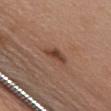{
  "biopsy_status": "not biopsied; imaged during a skin examination",
  "image": {
    "source": "total-body photography crop",
    "field_of_view_mm": 15
  },
  "site": "chest",
  "patient": {
    "sex": "male",
    "age_approx": 60
  },
  "automated_metrics": {
    "area_mm2_approx": 3.5,
    "eccentricity": 0.85,
    "shape_asymmetry": 0.4,
    "vs_skin_darker_L": 11.0,
    "vs_skin_contrast_norm": 8.5,
    "nevus_likeness_0_100": 75,
    "lesion_detection_confidence_0_100": 100
  },
  "lesion_size": {
    "long_diameter_mm_approx": 3.0
  }
}